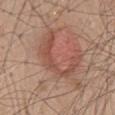Findings:
• biopsy status · no biopsy performed (imaged during a skin exam)
• site · the mid back
• acquisition · 15 mm crop, total-body photography
• patient · male, roughly 50 years of age